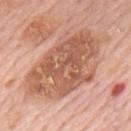biopsy status = total-body-photography surveillance lesion; no biopsy | lesion size = about 10 mm | image source = total-body-photography crop, ~15 mm field of view | patient = male, aged approximately 80 | tile lighting = white-light | body site = the mid back | image-analysis metrics = a classifier nevus-likeness of about 0/100 and a lesion-detection confidence of about 100/100.